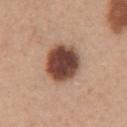| field | value |
|---|---|
| follow-up | imaged on a skin check; not biopsied |
| patient | female, aged approximately 30 |
| lighting | white-light illumination |
| image | total-body-photography crop, ~15 mm field of view |
| site | the chest |
| lesion size | ~5 mm (longest diameter) |